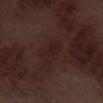follow-up: catalogued during a skin exam; not biopsied | lesion diameter: ~4 mm (longest diameter) | body site: the left thigh | image: ~15 mm tile from a whole-body skin photo | subject: male, roughly 70 years of age | lighting: white-light.